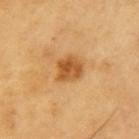Notes:
• notes: no biopsy performed (imaged during a skin exam)
• subject: male, aged around 60
• anatomic site: the left upper arm
• image: ~15 mm crop, total-body skin-cancer survey
• TBP lesion metrics: an average lesion color of about L≈54 a*≈24 b*≈44 (CIELAB)